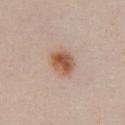The lesion was photographed on a routine skin check and not biopsied; there is no pathology result. From the chest. The subject is a female roughly 25 years of age. Cropped from a total-body skin-imaging series; the visible field is about 15 mm.